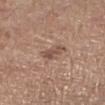| key | value |
|---|---|
| patient | male, in their mid-60s |
| site | the right forearm |
| size | ~3 mm (longest diameter) |
| image source | total-body-photography crop, ~15 mm field of view |
| image-analysis metrics | a border-irregularity index near 3/10 and a color-variation rating of about 3/10 |